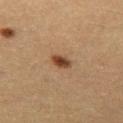{
  "biopsy_status": "not biopsied; imaged during a skin examination",
  "site": "left thigh",
  "patient": {
    "sex": "female",
    "age_approx": 30
  },
  "lighting": "cross-polarized",
  "lesion_size": {
    "long_diameter_mm_approx": 2.5
  },
  "image": {
    "source": "total-body photography crop",
    "field_of_view_mm": 15
  }
}